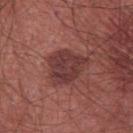Q: Is there a histopathology result?
A: imaged on a skin check; not biopsied
Q: What kind of image is this?
A: ~15 mm tile from a whole-body skin photo
Q: Lesion location?
A: the chest
Q: What are the patient's age and sex?
A: male, in their mid-60s